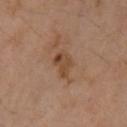follow-up: no biopsy performed (imaged during a skin exam)
acquisition: 15 mm crop, total-body photography
subject: female, about 45 years old
diameter: about 3.5 mm
location: the left upper arm
lighting: cross-polarized
automated metrics: a lesion area of about 5 mm², an eccentricity of roughly 0.8, and a symmetry-axis asymmetry near 0.35; a classifier nevus-likeness of about 15/100